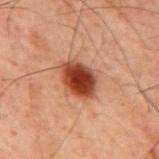No biopsy was performed on this lesion — it was imaged during a full skin examination and was not determined to be concerning. On the mid back. This is a cross-polarized tile. A close-up tile cropped from a whole-body skin photograph, about 15 mm across. The lesion's longest dimension is about 4.5 mm. A male subject roughly 60 years of age. The lesion-visualizer software estimated an outline eccentricity of about 0.7 (0 = round, 1 = elongated) and a shape-asymmetry score of about 0.15 (0 = symmetric). And it measured an average lesion color of about L≈34 a*≈23 b*≈28 (CIELAB) and roughly 15 lightness units darker than nearby skin. The software also gave border irregularity of about 1.5 on a 0–10 scale and a within-lesion color-variation index near 5.5/10. It also reported a nevus-likeness score of about 100/100 and lesion-presence confidence of about 100/100.